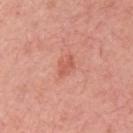Clinical impression: The lesion was tiled from a total-body skin photograph and was not biopsied. Acquisition and patient details: Measured at roughly 2.5 mm in maximum diameter. Located on the right upper arm. Imaged with white-light lighting. A region of skin cropped from a whole-body photographic capture, roughly 15 mm wide. A female patient aged approximately 65.